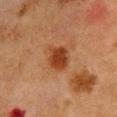Imaged during a routine full-body skin examination; the lesion was not biopsied and no histopathology is available. A roughly 15 mm field-of-view crop from a total-body skin photograph. Located on the chest. The patient is a female approximately 50 years of age. The total-body-photography lesion software estimated a lesion area of about 7 mm², a shape eccentricity near 0.55, and a symmetry-axis asymmetry near 0.2. The software also gave a lesion–skin lightness drop of about 10 and a lesion-to-skin contrast of about 10 (normalized; higher = more distinct). Imaged with cross-polarized lighting. The lesion's longest dimension is about 3 mm.